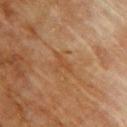Assessment: The lesion was photographed on a routine skin check and not biopsied; there is no pathology result. Acquisition and patient details: Located on the back. Captured under cross-polarized illumination. Automated image analysis of the tile measured a lesion area of about 3 mm², a shape eccentricity near 0.9, and two-axis asymmetry of about 0.45. The software also gave a mean CIELAB color near L≈41 a*≈20 b*≈33, about 5 CIELAB-L* units darker than the surrounding skin, and a lesion-to-skin contrast of about 5 (normalized; higher = more distinct). The subject is a female approximately 80 years of age. A roughly 15 mm field-of-view crop from a total-body skin photograph. Approximately 3 mm at its widest.